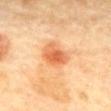– notes: catalogued during a skin exam; not biopsied
– automated lesion analysis: an average lesion color of about L≈68 a*≈31 b*≈45 (CIELAB), roughly 13 lightness units darker than nearby skin, and a normalized border contrast of about 7.5; a nevus-likeness score of about 100/100 and lesion-presence confidence of about 100/100
– patient: female, aged 58–62
– imaging modality: ~15 mm tile from a whole-body skin photo
– lesion size: about 3.5 mm
– anatomic site: the mid back
– tile lighting: cross-polarized illumination